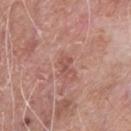This lesion was catalogued during total-body skin photography and was not selected for biopsy.
Located on the chest.
Imaged with white-light lighting.
A 15 mm close-up extracted from a 3D total-body photography capture.
A male patient roughly 65 years of age.
The recorded lesion diameter is about 2.5 mm.
Automated tile analysis of the lesion measured an average lesion color of about L≈52 a*≈24 b*≈25 (CIELAB), about 8 CIELAB-L* units darker than the surrounding skin, and a normalized lesion–skin contrast near 5.5. It also reported border irregularity of about 3.5 on a 0–10 scale, a within-lesion color-variation index near 0.5/10, and a peripheral color-asymmetry measure near 0. It also reported an automated nevus-likeness rating near 0 out of 100 and lesion-presence confidence of about 100/100.